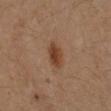<record>
  <biopsy_status>not biopsied; imaged during a skin examination</biopsy_status>
  <site>left forearm</site>
  <lesion_size>
    <long_diameter_mm_approx>3.0</long_diameter_mm_approx>
  </lesion_size>
  <image>
    <source>total-body photography crop</source>
    <field_of_view_mm>15</field_of_view_mm>
  </image>
  <patient>
    <sex>female</sex>
    <age_approx>30</age_approx>
  </patient>
</record>